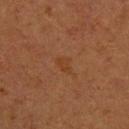This lesion was catalogued during total-body skin photography and was not selected for biopsy.
Imaged with cross-polarized lighting.
A roughly 15 mm field-of-view crop from a total-body skin photograph.
On the upper back.
A female subject in their 40s.